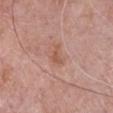biopsy_status: not biopsied; imaged during a skin examination
patient:
  sex: male
  age_approx: 85+
site: chest
image:
  source: total-body photography crop
  field_of_view_mm: 15